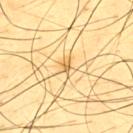Q: Is there a histopathology result?
A: catalogued during a skin exam; not biopsied
Q: Automated lesion metrics?
A: a footprint of about 1.5 mm², a shape eccentricity near 0.65, and two-axis asymmetry of about 0.3; an average lesion color of about L≈67 a*≈17 b*≈46 (CIELAB), a lesion–skin lightness drop of about 13, and a lesion-to-skin contrast of about 7.5 (normalized; higher = more distinct); border irregularity of about 2 on a 0–10 scale, internal color variation of about 0 on a 0–10 scale, and radial color variation of about 0
Q: Where on the body is the lesion?
A: the upper back
Q: Who is the patient?
A: male, aged 43 to 47
Q: How was this image acquired?
A: total-body-photography crop, ~15 mm field of view